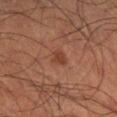– notes — total-body-photography surveillance lesion; no biopsy
– illumination — cross-polarized
– patient — male, aged approximately 35
– image — ~15 mm crop, total-body skin-cancer survey
– site — the right lower leg